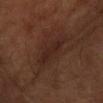Clinical impression: Captured during whole-body skin photography for melanoma surveillance; the lesion was not biopsied. Clinical summary: Measured at roughly 6 mm in maximum diameter. On the left forearm. A 15 mm crop from a total-body photograph taken for skin-cancer surveillance. A male subject, aged approximately 60. This is a cross-polarized tile.